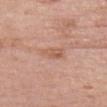Impression: The lesion was photographed on a routine skin check and not biopsied; there is no pathology result.